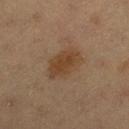Assessment: Recorded during total-body skin imaging; not selected for excision or biopsy. Acquisition and patient details: The lesion is located on the leg. Captured under cross-polarized illumination. The patient is a female roughly 20 years of age. About 4.5 mm across. Cropped from a total-body skin-imaging series; the visible field is about 15 mm. Automated tile analysis of the lesion measured an average lesion color of about L≈36 a*≈15 b*≈27 (CIELAB) and about 7 CIELAB-L* units darker than the surrounding skin.